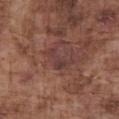| feature | finding |
|---|---|
| follow-up | no biopsy performed (imaged during a skin exam) |
| image | ~15 mm tile from a whole-body skin photo |
| patient | male, aged approximately 75 |
| tile lighting | white-light |
| diameter | about 4 mm |
| anatomic site | the chest |